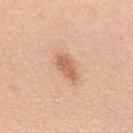Notes:
* subject · male, aged 48–52
* lesion diameter · ~3.5 mm (longest diameter)
* location · the mid back
* automated metrics · a footprint of about 4.5 mm² and an eccentricity of roughly 0.85; border irregularity of about 3 on a 0–10 scale, a color-variation rating of about 2/10, and peripheral color asymmetry of about 0.5
* lighting · white-light
* acquisition · ~15 mm tile from a whole-body skin photo